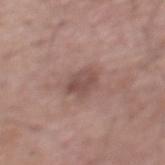* follow-up · total-body-photography surveillance lesion; no biopsy
* diameter · ~3 mm (longest diameter)
* subject · male, aged approximately 55
* image · total-body-photography crop, ~15 mm field of view
* lighting · white-light
* location · the mid back